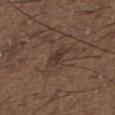follow-up: imaged on a skin check; not biopsied | lesion diameter: ≈3 mm | automated lesion analysis: internal color variation of about 2 on a 0–10 scale and radial color variation of about 0.5 | lighting: white-light illumination | patient: male, aged approximately 50 | anatomic site: the upper back | image: ~15 mm crop, total-body skin-cancer survey.